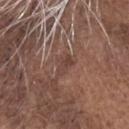Q: Was this lesion biopsied?
A: total-body-photography surveillance lesion; no biopsy
Q: What lighting was used for the tile?
A: white-light
Q: Lesion location?
A: the head or neck
Q: Patient demographics?
A: male, approximately 75 years of age
Q: What is the lesion's diameter?
A: about 3 mm
Q: What kind of image is this?
A: ~15 mm tile from a whole-body skin photo